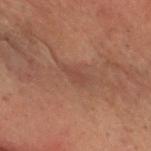The lesion was photographed on a routine skin check and not biopsied; there is no pathology result.
The lesion is on the head or neck.
Automated tile analysis of the lesion measured a footprint of about 3 mm², an eccentricity of roughly 0.9, and two-axis asymmetry of about 0.35. And it measured a mean CIELAB color near L≈33 a*≈17 b*≈22 and about 5 CIELAB-L* units darker than the surrounding skin. It also reported a border-irregularity index near 3.5/10 and a within-lesion color-variation index near 0.5/10. It also reported an automated nevus-likeness rating near 0 out of 100 and a detector confidence of about 65 out of 100 that the crop contains a lesion.
This is a cross-polarized tile.
The subject is a male aged around 70.
About 2.5 mm across.
Cropped from a total-body skin-imaging series; the visible field is about 15 mm.